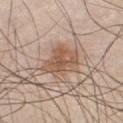| key | value |
|---|---|
| biopsy status | imaged on a skin check; not biopsied |